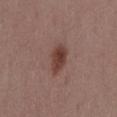Clinical impression: The lesion was photographed on a routine skin check and not biopsied; there is no pathology result. Background: Cropped from a total-body skin-imaging series; the visible field is about 15 mm. The lesion-visualizer software estimated a lesion color around L≈41 a*≈20 b*≈23 in CIELAB, a lesion–skin lightness drop of about 10, and a normalized lesion–skin contrast near 9. The software also gave a border-irregularity index near 1.5/10, a within-lesion color-variation index near 3.5/10, and a peripheral color-asymmetry measure near 1. It also reported a nevus-likeness score of about 100/100 and a detector confidence of about 100 out of 100 that the crop contains a lesion. This is a white-light tile. About 4 mm across. The lesion is located on the lower back. A female subject, aged approximately 55.